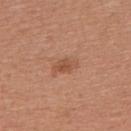biopsy status: catalogued during a skin exam; not biopsied